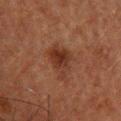Q: Is there a histopathology result?
A: imaged on a skin check; not biopsied